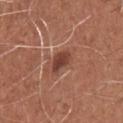biopsy_status: not biopsied; imaged during a skin examination
site: chest
image:
  source: total-body photography crop
  field_of_view_mm: 15
patient:
  sex: male
  age_approx: 65
lighting: white-light